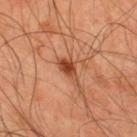Notes:
• subject: male, in their mid-40s
• image source: total-body-photography crop, ~15 mm field of view
• tile lighting: cross-polarized illumination
• lesion size: about 3.5 mm
• automated metrics: border irregularity of about 3 on a 0–10 scale and internal color variation of about 3 on a 0–10 scale; a nevus-likeness score of about 95/100 and a lesion-detection confidence of about 100/100
• anatomic site: the back